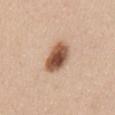The lesion was tiled from a total-body skin photograph and was not biopsied. Located on the abdomen. A region of skin cropped from a whole-body photographic capture, roughly 15 mm wide. A male patient, approximately 40 years of age.